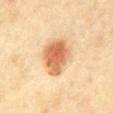Findings:
– imaging modality: 15 mm crop, total-body photography
– patient: male, about 70 years old
– body site: the abdomen
– automated metrics: a shape eccentricity near 0.6 and a symmetry-axis asymmetry near 0.2; a mean CIELAB color near L≈63 a*≈22 b*≈38, roughly 15 lightness units darker than nearby skin, and a normalized lesion–skin contrast near 9; a border-irregularity index near 2/10 and a peripheral color-asymmetry measure near 1.5; a lesion-detection confidence of about 100/100